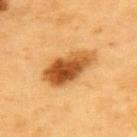| field | value |
|---|---|
| image | ~15 mm crop, total-body skin-cancer survey |
| subject | male, aged 58–62 |
| location | the upper back |
| tile lighting | cross-polarized |